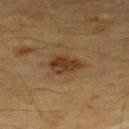workup: total-body-photography surveillance lesion; no biopsy
body site: the upper back
acquisition: ~15 mm crop, total-body skin-cancer survey
lesion size: ≈4 mm
patient: male, approximately 55 years of age